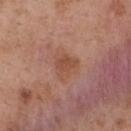notes=total-body-photography surveillance lesion; no biopsy | anatomic site=the upper back | subject=female, aged around 40 | imaging modality=total-body-photography crop, ~15 mm field of view.